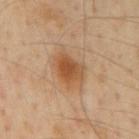Clinical impression:
This lesion was catalogued during total-body skin photography and was not selected for biopsy.
Context:
The lesion is on the mid back. This is a cross-polarized tile. A male patient aged approximately 55. Automated image analysis of the tile measured a lesion area of about 9 mm² and two-axis asymmetry of about 0.25. It also reported a mean CIELAB color near L≈52 a*≈22 b*≈37 and roughly 11 lightness units darker than nearby skin. The analysis additionally found border irregularity of about 2.5 on a 0–10 scale and radial color variation of about 1. Cropped from a total-body skin-imaging series; the visible field is about 15 mm.